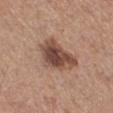Clinical impression: Imaged during a routine full-body skin examination; the lesion was not biopsied and no histopathology is available. Context: This is a white-light tile. Cropped from a whole-body photographic skin survey; the tile spans about 15 mm. Approximately 5.5 mm at its widest. The patient is a male roughly 75 years of age. The lesion is on the right lower leg. The total-body-photography lesion software estimated a border-irregularity rating of about 3.5/10 and a within-lesion color-variation index near 5.5/10.